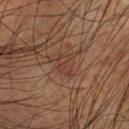Cropped from a total-body skin-imaging series; the visible field is about 15 mm.
A male subject aged around 60.
Captured under cross-polarized illumination.
Measured at roughly 3 mm in maximum diameter.
On the left forearm.